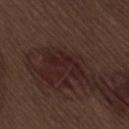Captured during whole-body skin photography for melanoma surveillance; the lesion was not biopsied. A close-up tile cropped from a whole-body skin photograph, about 15 mm across. From the lower back. Measured at roughly 5 mm in maximum diameter. A male patient aged around 70. This is a white-light tile.